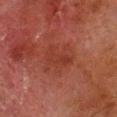Recorded during total-body skin imaging; not selected for excision or biopsy. A male subject aged 78–82. This is a cross-polarized tile. The lesion is on the leg. About 4 mm across. An algorithmic analysis of the crop reported a footprint of about 11 mm² and two-axis asymmetry of about 0.3. It also reported a lesion color around L≈30 a*≈23 b*≈25 in CIELAB, about 4 CIELAB-L* units darker than the surrounding skin, and a normalized border contrast of about 5. A region of skin cropped from a whole-body photographic capture, roughly 15 mm wide.